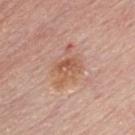This lesion was catalogued during total-body skin photography and was not selected for biopsy. A close-up tile cropped from a whole-body skin photograph, about 15 mm across. Measured at roughly 5 mm in maximum diameter. The patient is a male aged approximately 60. An algorithmic analysis of the crop reported a footprint of about 12 mm², an eccentricity of roughly 0.7, and a shape-asymmetry score of about 0.3 (0 = symmetric). It also reported a lesion color around L≈58 a*≈22 b*≈31 in CIELAB. The analysis additionally found a color-variation rating of about 4.5/10 and peripheral color asymmetry of about 1.5. The tile uses white-light illumination. On the chest.